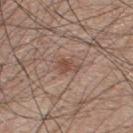automated metrics: roughly 9 lightness units darker than nearby skin and a lesion-to-skin contrast of about 7 (normalized; higher = more distinct); a nevus-likeness score of about 0/100 and a detector confidence of about 100 out of 100 that the crop contains a lesion
site: the upper back
image: 15 mm crop, total-body photography
illumination: white-light
patient: male, roughly 50 years of age